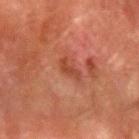The lesion was photographed on a routine skin check and not biopsied; there is no pathology result. Imaged with cross-polarized lighting. The lesion-visualizer software estimated a lesion area of about 3.5 mm² and a shape-asymmetry score of about 0.35 (0 = symmetric). And it measured a color-variation rating of about 0/10 and peripheral color asymmetry of about 0. The subject is a male aged 78 to 82. A close-up tile cropped from a whole-body skin photograph, about 15 mm across. From the arm.